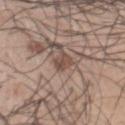On the chest.
Cropped from a whole-body photographic skin survey; the tile spans about 15 mm.
A male subject roughly 55 years of age.